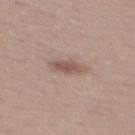No biopsy was performed on this lesion — it was imaged during a full skin examination and was not determined to be concerning.
A female subject aged approximately 25.
A 15 mm crop from a total-body photograph taken for skin-cancer surveillance.
On the mid back.
The total-body-photography lesion software estimated a lesion area of about 5 mm², an eccentricity of roughly 0.9, and a shape-asymmetry score of about 0.15 (0 = symmetric). The analysis additionally found border irregularity of about 2 on a 0–10 scale, internal color variation of about 2.5 on a 0–10 scale, and peripheral color asymmetry of about 1. The software also gave a nevus-likeness score of about 40/100 and a detector confidence of about 100 out of 100 that the crop contains a lesion.
This is a white-light tile.
The lesion's longest dimension is about 4 mm.